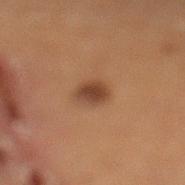This lesion was catalogued during total-body skin photography and was not selected for biopsy. The tile uses cross-polarized illumination. On the left lower leg. The lesion's longest dimension is about 2.5 mm. A close-up tile cropped from a whole-body skin photograph, about 15 mm across. The subject is a male aged 58–62.